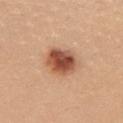Q: Lesion location?
A: the upper back
Q: What did automated image analysis measure?
A: lesion-presence confidence of about 100/100
Q: Who is the patient?
A: female, in their mid- to late 20s
Q: How was this image acquired?
A: ~15 mm tile from a whole-body skin photo
Q: What is the lesion's diameter?
A: ~4.5 mm (longest diameter)
Q: Illumination type?
A: white-light